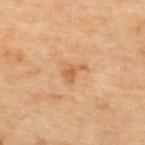Image and clinical context: A female subject, aged around 65. A roughly 15 mm field-of-view crop from a total-body skin photograph. This is a cross-polarized tile. The lesion is on the upper back. The recorded lesion diameter is about 2.5 mm.